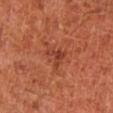Case summary:
• workup · total-body-photography surveillance lesion; no biopsy
• subject · male, in their mid- to late 60s
• lesion size · ≈2.5 mm
• site · the left lower leg
• acquisition · ~15 mm tile from a whole-body skin photo
• tile lighting · cross-polarized illumination